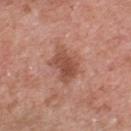Q: Was a biopsy performed?
A: catalogued during a skin exam; not biopsied
Q: What did automated image analysis measure?
A: a footprint of about 10 mm², a shape eccentricity near 0.75, and two-axis asymmetry of about 0.3; an average lesion color of about L≈51 a*≈24 b*≈29 (CIELAB) and a lesion-to-skin contrast of about 7.5 (normalized; higher = more distinct); a border-irregularity index near 3.5/10, a within-lesion color-variation index near 3/10, and radial color variation of about 1
Q: What kind of image is this?
A: ~15 mm tile from a whole-body skin photo
Q: Who is the patient?
A: male, in their 70s
Q: How was the tile lit?
A: white-light illumination
Q: Lesion size?
A: about 4.5 mm
Q: Lesion location?
A: the front of the torso